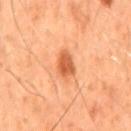| field | value |
|---|---|
| anatomic site | the mid back |
| illumination | cross-polarized illumination |
| diameter | ≈3.5 mm |
| patient | male, aged 48–52 |
| image source | ~15 mm tile from a whole-body skin photo |